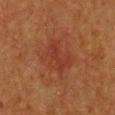Clinical impression: The lesion was photographed on a routine skin check and not biopsied; there is no pathology result. Image and clinical context: Cropped from a whole-body photographic skin survey; the tile spans about 15 mm. Approximately 4.5 mm at its widest. A female subject aged 38 to 42. Located on the chest. An algorithmic analysis of the crop reported a shape eccentricity near 0.65 and a shape-asymmetry score of about 0.3 (0 = symmetric). The software also gave roughly 5 lightness units darker than nearby skin and a normalized border contrast of about 5. It also reported a classifier nevus-likeness of about 0/100. The tile uses cross-polarized illumination.